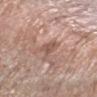| feature | finding |
|---|---|
| notes | catalogued during a skin exam; not biopsied |
| illumination | white-light illumination |
| subject | male, about 60 years old |
| acquisition | total-body-photography crop, ~15 mm field of view |
| location | the left forearm |
| diameter | about 2.5 mm |